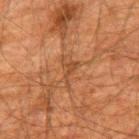{"biopsy_status": "not biopsied; imaged during a skin examination", "lighting": "cross-polarized", "patient": {"sex": "male", "age_approx": 60}, "site": "upper back", "automated_metrics": {"eccentricity": 0.85, "shape_asymmetry": 0.65, "cielab_L": 36, "cielab_a": 19, "cielab_b": 29, "vs_skin_darker_L": 6.0, "vs_skin_contrast_norm": 5.5, "border_irregularity_0_10": 8.5, "color_variation_0_10": 0.5, "peripheral_color_asymmetry": 0.0, "nevus_likeness_0_100": 0}, "image": {"source": "total-body photography crop", "field_of_view_mm": 15}}